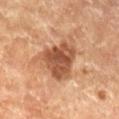This lesion was catalogued during total-body skin photography and was not selected for biopsy.
The lesion-visualizer software estimated an average lesion color of about L≈51 a*≈23 b*≈33 (CIELAB), roughly 13 lightness units darker than nearby skin, and a normalized lesion–skin contrast near 9. The analysis additionally found border irregularity of about 5 on a 0–10 scale, internal color variation of about 4.5 on a 0–10 scale, and peripheral color asymmetry of about 1.5.
The lesion is on the left lower leg.
The tile uses cross-polarized illumination.
Measured at roughly 6 mm in maximum diameter.
A 15 mm close-up extracted from a 3D total-body photography capture.
The subject is a female aged around 70.